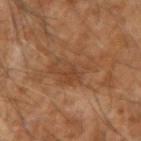No biopsy was performed on this lesion — it was imaged during a full skin examination and was not determined to be concerning.
Located on the right forearm.
A 15 mm close-up tile from a total-body photography series done for melanoma screening.
Imaged with cross-polarized lighting.
The lesion's longest dimension is about 5 mm.
The patient is a male in their mid-50s.